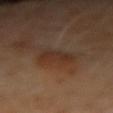Q: Was this lesion biopsied?
A: no biopsy performed (imaged during a skin exam)
Q: How was the tile lit?
A: cross-polarized
Q: Where on the body is the lesion?
A: the arm
Q: What is the lesion's diameter?
A: ~4 mm (longest diameter)
Q: What kind of image is this?
A: ~15 mm tile from a whole-body skin photo
Q: Patient demographics?
A: female, roughly 50 years of age